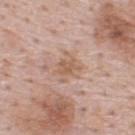Image and clinical context: A male patient aged 48–52. This is a white-light tile. The lesion's longest dimension is about 3 mm. A 15 mm crop from a total-body photograph taken for skin-cancer surveillance. On the upper back.